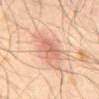<case>
  <biopsy_status>not biopsied; imaged during a skin examination</biopsy_status>
</case>